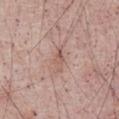Part of a total-body skin-imaging series; this lesion was reviewed on a skin check and was not flagged for biopsy. Automated tile analysis of the lesion measured a lesion–skin lightness drop of about 8 and a normalized lesion–skin contrast near 5.5. And it measured an automated nevus-likeness rating near 0 out of 100 and a detector confidence of about 100 out of 100 that the crop contains a lesion. Imaged with white-light lighting. A male patient, aged approximately 55. From the abdomen. This image is a 15 mm lesion crop taken from a total-body photograph. The lesion's longest dimension is about 3 mm.